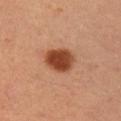The lesion was tiled from a total-body skin photograph and was not biopsied. Captured under cross-polarized illumination. The lesion-visualizer software estimated an area of roughly 10 mm² and a shape eccentricity near 0.2. The analysis additionally found an average lesion color of about L≈43 a*≈26 b*≈33 (CIELAB), roughly 16 lightness units darker than nearby skin, and a normalized lesion–skin contrast near 11.5. A region of skin cropped from a whole-body photographic capture, roughly 15 mm wide. The lesion is located on the right upper arm. The subject is a male roughly 35 years of age. About 3.5 mm across.